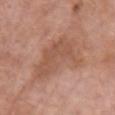Recorded during total-body skin imaging; not selected for excision or biopsy. A male patient aged around 80. This image is a 15 mm lesion crop taken from a total-body photograph. Automated tile analysis of the lesion measured a nevus-likeness score of about 0/100 and a lesion-detection confidence of about 100/100. About 7 mm across. From the front of the torso.